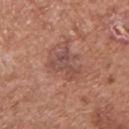Impression: The lesion was tiled from a total-body skin photograph and was not biopsied. Context: Located on the chest. Imaged with white-light lighting. This image is a 15 mm lesion crop taken from a total-body photograph. The recorded lesion diameter is about 3.5 mm. A male patient in their mid-70s. Automated image analysis of the tile measured a lesion color around L≈49 a*≈22 b*≈25 in CIELAB and a normalized lesion–skin contrast near 6. And it measured a border-irregularity index near 6.5/10 and a color-variation rating of about 4/10.